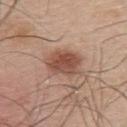notes = no biopsy performed (imaged during a skin exam) | tile lighting = white-light illumination | imaging modality = ~15 mm tile from a whole-body skin photo | patient = male, approximately 45 years of age | lesion size = ≈4 mm | anatomic site = the back.